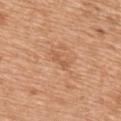workup: catalogued during a skin exam; not biopsied
location: the upper back
patient: female, in their mid- to late 50s
image source: 15 mm crop, total-body photography
automated metrics: an area of roughly 2.5 mm², an outline eccentricity of about 0.95 (0 = round, 1 = elongated), and two-axis asymmetry of about 0.25; internal color variation of about 0 on a 0–10 scale